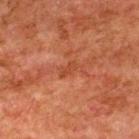This lesion was catalogued during total-body skin photography and was not selected for biopsy. A male patient aged 78 to 82. The lesion is located on the upper back. The lesion-visualizer software estimated a footprint of about 3 mm², an outline eccentricity of about 0.85 (0 = round, 1 = elongated), and a shape-asymmetry score of about 0.4 (0 = symmetric). The software also gave a lesion color around L≈36 a*≈25 b*≈30 in CIELAB and a normalized border contrast of about 5. And it measured a border-irregularity index near 4/10 and a peripheral color-asymmetry measure near 0. A 15 mm close-up extracted from a 3D total-body photography capture. The lesion's longest dimension is about 3 mm.